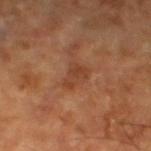image = total-body-photography crop, ~15 mm field of view
tile lighting = cross-polarized illumination
subject = male, aged approximately 65
anatomic site = the left lower leg